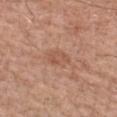Imaged during a routine full-body skin examination; the lesion was not biopsied and no histopathology is available.
Imaged with white-light lighting.
Approximately 3 mm at its widest.
A lesion tile, about 15 mm wide, cut from a 3D total-body photograph.
A male subject about 60 years old.
The lesion is on the arm.